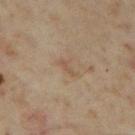Case summary:
– follow-up: imaged on a skin check; not biopsied
– tile lighting: cross-polarized illumination
– size: ~2.5 mm (longest diameter)
– imaging modality: ~15 mm crop, total-body skin-cancer survey
– site: the left thigh
– subject: female, aged around 35